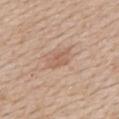Imaged during a routine full-body skin examination; the lesion was not biopsied and no histopathology is available.
About 3 mm across.
Automated tile analysis of the lesion measured an area of roughly 4 mm² and a shape-asymmetry score of about 0.35 (0 = symmetric).
From the mid back.
A 15 mm close-up extracted from a 3D total-body photography capture.
The patient is a male aged around 60.